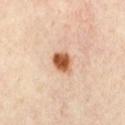Imaged during a routine full-body skin examination; the lesion was not biopsied and no histopathology is available. The subject is aged 53–57. The lesion is located on the chest. Approximately 3 mm at its widest. Automated image analysis of the tile measured a shape eccentricity near 0.6 and two-axis asymmetry of about 0.15. And it measured a mean CIELAB color near L≈57 a*≈25 b*≈36, roughly 18 lightness units darker than nearby skin, and a normalized lesion–skin contrast near 12. A close-up tile cropped from a whole-body skin photograph, about 15 mm across.